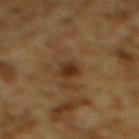Assessment:
The lesion was photographed on a routine skin check and not biopsied; there is no pathology result.
Acquisition and patient details:
On the upper back. An algorithmic analysis of the crop reported an average lesion color of about L≈27 a*≈16 b*≈27 (CIELAB), a lesion–skin lightness drop of about 9, and a normalized border contrast of about 9. And it measured a border-irregularity index near 2.5/10, internal color variation of about 1.5 on a 0–10 scale, and radial color variation of about 0.5. It also reported a nevus-likeness score of about 35/100 and lesion-presence confidence of about 100/100. A male subject, in their mid-80s or older. A 15 mm close-up extracted from a 3D total-body photography capture. The tile uses cross-polarized illumination. Approximately 3 mm at its widest.